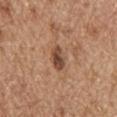Clinical summary:
This is a white-light tile. The lesion is located on the head or neck. Approximately 3 mm at its widest. Cropped from a total-body skin-imaging series; the visible field is about 15 mm. A male patient approximately 70 years of age. The lesion-visualizer software estimated a footprint of about 5 mm², an outline eccentricity of about 0.8 (0 = round, 1 = elongated), and a shape-asymmetry score of about 0.25 (0 = symmetric). The analysis additionally found a classifier nevus-likeness of about 65/100 and lesion-presence confidence of about 100/100.